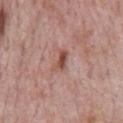follow-up: total-body-photography surveillance lesion; no biopsy
TBP lesion metrics: an average lesion color of about L≈50 a*≈23 b*≈26 (CIELAB); border irregularity of about 3.5 on a 0–10 scale and radial color variation of about 1
patient: male, aged 68 to 72
image: total-body-photography crop, ~15 mm field of view
location: the chest
diameter: ~3 mm (longest diameter)